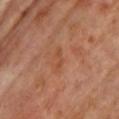<tbp_lesion>
<biopsy_status>not biopsied; imaged during a skin examination</biopsy_status>
<lighting>cross-polarized</lighting>
<patient>
  <sex>female</sex>
  <age_approx>65</age_approx>
</patient>
<image>
  <source>total-body photography crop</source>
  <field_of_view_mm>15</field_of_view_mm>
</image>
<lesion_size>
  <long_diameter_mm_approx>3.0</long_diameter_mm_approx>
</lesion_size>
<site>chest</site>
</tbp_lesion>